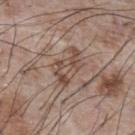Q: Is there a histopathology result?
A: catalogued during a skin exam; not biopsied